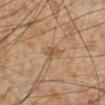{
  "biopsy_status": "not biopsied; imaged during a skin examination",
  "patient": {
    "sex": "male",
    "age_approx": 55
  },
  "image": {
    "source": "total-body photography crop",
    "field_of_view_mm": 15
  },
  "site": "left lower leg"
}